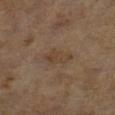Imaged during a routine full-body skin examination; the lesion was not biopsied and no histopathology is available.
A 15 mm close-up tile from a total-body photography series done for melanoma screening.
From the left lower leg.
About 3.5 mm across.
A female subject, roughly 60 years of age.
This is a cross-polarized tile.